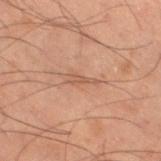Q: Was this lesion biopsied?
A: catalogued during a skin exam; not biopsied
Q: What is the imaging modality?
A: ~15 mm crop, total-body skin-cancer survey
Q: What are the patient's age and sex?
A: male, in their 60s
Q: What is the anatomic site?
A: the leg